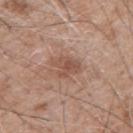Captured during whole-body skin photography for melanoma surveillance; the lesion was not biopsied. On the right upper arm. A 15 mm close-up tile from a total-body photography series done for melanoma screening. The lesion's longest dimension is about 3.5 mm. The total-body-photography lesion software estimated an eccentricity of roughly 0.7 and a symmetry-axis asymmetry near 0.35. And it measured a mean CIELAB color near L≈51 a*≈21 b*≈27, about 9 CIELAB-L* units darker than the surrounding skin, and a normalized lesion–skin contrast near 6.5. And it measured border irregularity of about 4 on a 0–10 scale, a color-variation rating of about 2.5/10, and peripheral color asymmetry of about 1. Captured under white-light illumination. A male subject, aged approximately 60.